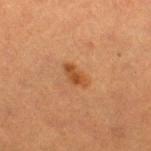Q: Was a biopsy performed?
A: no biopsy performed (imaged during a skin exam)
Q: Automated lesion metrics?
A: an area of roughly 4 mm² and two-axis asymmetry of about 0.25; an average lesion color of about L≈42 a*≈22 b*≈35 (CIELAB), about 9 CIELAB-L* units darker than the surrounding skin, and a normalized border contrast of about 7.5
Q: What is the anatomic site?
A: the right thigh
Q: What are the patient's age and sex?
A: female, in their mid-50s
Q: How was this image acquired?
A: total-body-photography crop, ~15 mm field of view
Q: What lighting was used for the tile?
A: cross-polarized illumination